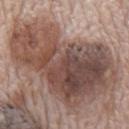Impression: The lesion was photographed on a routine skin check and not biopsied; there is no pathology result. Context: Located on the mid back. A male subject about 70 years old. Longest diameter approximately 14 mm. Cropped from a total-body skin-imaging series; the visible field is about 15 mm.